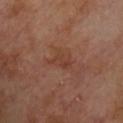biopsy status: imaged on a skin check; not biopsied | location: the chest | image source: total-body-photography crop, ~15 mm field of view | patient: male, aged 68 to 72.